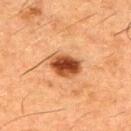{"biopsy_status": "not biopsied; imaged during a skin examination", "lesion_size": {"long_diameter_mm_approx": 4.0}, "image": {"source": "total-body photography crop", "field_of_view_mm": 15}, "patient": {"sex": "male", "age_approx": 50}, "site": "back", "lighting": "cross-polarized"}